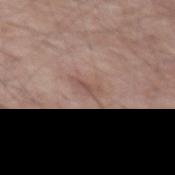Case summary:
* follow-up — imaged on a skin check; not biopsied
* image — ~15 mm crop, total-body skin-cancer survey
* subject — male, approximately 65 years of age
* lesion diameter — ~2.5 mm (longest diameter)
* lighting — white-light illumination
* anatomic site — the left thigh